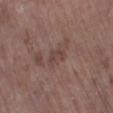Q: Was a biopsy performed?
A: catalogued during a skin exam; not biopsied
Q: How was this image acquired?
A: ~15 mm crop, total-body skin-cancer survey
Q: Patient demographics?
A: female, in their mid-80s or older
Q: Where on the body is the lesion?
A: the left lower leg
Q: Lesion size?
A: about 3 mm
Q: Automated lesion metrics?
A: a shape eccentricity near 0.8 and two-axis asymmetry of about 0.3; a border-irregularity rating of about 3/10, a color-variation rating of about 2.5/10, and a peripheral color-asymmetry measure near 1; a nevus-likeness score of about 0/100 and lesion-presence confidence of about 95/100
Q: How was the tile lit?
A: white-light